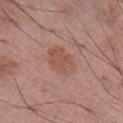Part of a total-body skin-imaging series; this lesion was reviewed on a skin check and was not flagged for biopsy.
Longest diameter approximately 4 mm.
A region of skin cropped from a whole-body photographic capture, roughly 15 mm wide.
Imaged with white-light lighting.
The subject is a male about 70 years old.
The lesion is on the right lower leg.